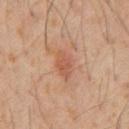| key | value |
|---|---|
| site | the abdomen |
| image-analysis metrics | a mean CIELAB color near L≈51 a*≈22 b*≈30, a lesion–skin lightness drop of about 7, and a normalized border contrast of about 5.5; a border-irregularity index near 2.5/10, a within-lesion color-variation index near 2.5/10, and radial color variation of about 1 |
| tile lighting | cross-polarized illumination |
| size | ≈3.5 mm |
| acquisition | ~15 mm tile from a whole-body skin photo |
| subject | male, approximately 60 years of age |